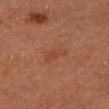Notes:
• workup · catalogued during a skin exam; not biopsied
• size · ≈3.5 mm
• image source · total-body-photography crop, ~15 mm field of view
• body site · the head or neck
• patient · female, aged 68–72